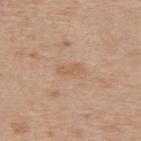site = the back
illumination = white-light illumination
subject = male, roughly 70 years of age
automated lesion analysis = a lesion color around L≈59 a*≈18 b*≈33 in CIELAB, roughly 6 lightness units darker than nearby skin, and a normalized border contrast of about 5; a detector confidence of about 100 out of 100 that the crop contains a lesion
image source = ~15 mm tile from a whole-body skin photo
lesion diameter = ~3 mm (longest diameter)